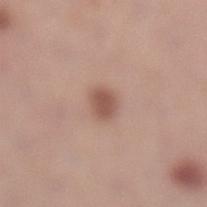An algorithmic analysis of the crop reported roughly 11 lightness units darker than nearby skin and a normalized border contrast of about 7.5. It also reported a border-irregularity index near 1.5/10, a within-lesion color-variation index near 2/10, and a peripheral color-asymmetry measure near 0.5. The analysis additionally found a classifier nevus-likeness of about 95/100 and lesion-presence confidence of about 100/100.
A female patient, in their 30s.
A 15 mm close-up extracted from a 3D total-body photography capture.
On the leg.